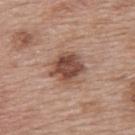Acquisition and patient details:
On the upper back. The tile uses white-light illumination. Measured at roughly 4 mm in maximum diameter. A female subject, aged 58 to 62. Automated image analysis of the tile measured an average lesion color of about L≈47 a*≈21 b*≈27 (CIELAB), a lesion–skin lightness drop of about 14, and a normalized lesion–skin contrast near 10. And it measured border irregularity of about 2 on a 0–10 scale. The analysis additionally found a classifier nevus-likeness of about 65/100 and a lesion-detection confidence of about 100/100. Cropped from a whole-body photographic skin survey; the tile spans about 15 mm.